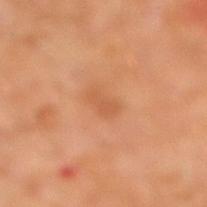<lesion>
  <patient>
    <sex>male</sex>
    <age_approx>30</age_approx>
  </patient>
  <lesion_size>
    <long_diameter_mm_approx>2.5</long_diameter_mm_approx>
  </lesion_size>
  <site>left leg</site>
  <automated_metrics>
    <area_mm2_approx>3.5</area_mm2_approx>
    <shape_asymmetry>0.3</shape_asymmetry>
    <vs_skin_darker_L>7.0</vs_skin_darker_L>
    <vs_skin_contrast_norm>5.0</vs_skin_contrast_norm>
    <nevus_likeness_0_100>5</nevus_likeness_0_100>
    <lesion_detection_confidence_0_100>100</lesion_detection_confidence_0_100>
  </automated_metrics>
  <lighting>cross-polarized</lighting>
  <image>
    <source>total-body photography crop</source>
    <field_of_view_mm>15</field_of_view_mm>
  </image>
</lesion>